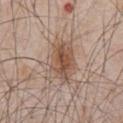Notes:
– anatomic site · the chest
– image source · 15 mm crop, total-body photography
– illumination · white-light illumination
– TBP lesion metrics · roughly 10 lightness units darker than nearby skin and a lesion-to-skin contrast of about 7.5 (normalized; higher = more distinct); a border-irregularity index near 3/10, a within-lesion color-variation index near 7/10, and peripheral color asymmetry of about 2.5
– patient · male, in their mid-60s